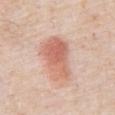- follow-up — no biopsy performed (imaged during a skin exam)
- automated lesion analysis — a footprint of about 17 mm², an eccentricity of roughly 0.85, and two-axis asymmetry of about 0.3; a lesion color around L≈64 a*≈24 b*≈30 in CIELAB, about 11 CIELAB-L* units darker than the surrounding skin, and a lesion-to-skin contrast of about 7 (normalized; higher = more distinct)
- subject — male, about 80 years old
- body site — the abdomen
- diameter — ≈6 mm
- imaging modality — 15 mm crop, total-body photography
- lighting — white-light